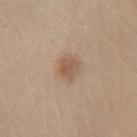<lesion>
<biopsy_status>not biopsied; imaged during a skin examination</biopsy_status>
<image>
  <source>total-body photography crop</source>
  <field_of_view_mm>15</field_of_view_mm>
</image>
<patient>
  <sex>female</sex>
  <age_approx>50</age_approx>
</patient>
<automated_metrics>
  <area_mm2_approx>5.0</area_mm2_approx>
  <eccentricity>0.4</eccentricity>
  <shape_asymmetry>0.25</shape_asymmetry>
  <cielab_L>45</cielab_L>
  <cielab_a>14</cielab_a>
  <cielab_b>25</cielab_b>
  <border_irregularity_0_10>2.5</border_irregularity_0_10>
  <peripheral_color_asymmetry>0.5</peripheral_color_asymmetry>
</automated_metrics>
<site>leg</site>
<lesion_size>
  <long_diameter_mm_approx>2.5</long_diameter_mm_approx>
</lesion_size>
<lighting>cross-polarized</lighting>
</lesion>